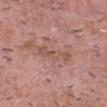Captured during whole-body skin photography for melanoma surveillance; the lesion was not biopsied. A 15 mm close-up extracted from a 3D total-body photography capture. Imaged with white-light lighting. A male subject, aged 53 to 57. Automated tile analysis of the lesion measured a lesion area of about 6 mm² and an outline eccentricity of about 0.95 (0 = round, 1 = elongated). And it measured an average lesion color of about L≈53 a*≈22 b*≈26 (CIELAB), about 7 CIELAB-L* units darker than the surrounding skin, and a normalized lesion–skin contrast near 5.5. It also reported a within-lesion color-variation index near 2/10. Located on the head or neck. The recorded lesion diameter is about 4.5 mm.